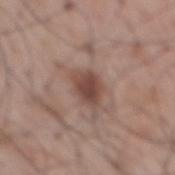workup: no biopsy performed (imaged during a skin exam)
acquisition: 15 mm crop, total-body photography
subject: male, roughly 70 years of age
site: the mid back